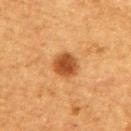Imaged with cross-polarized lighting. The patient is a male in their mid-70s. From the upper back. A close-up tile cropped from a whole-body skin photograph, about 15 mm across. An algorithmic analysis of the crop reported a footprint of about 7 mm², an eccentricity of roughly 0.6, and a symmetry-axis asymmetry near 0.15. And it measured border irregularity of about 1 on a 0–10 scale, a within-lesion color-variation index near 3.5/10, and peripheral color asymmetry of about 1. It also reported an automated nevus-likeness rating near 100 out of 100 and a detector confidence of about 100 out of 100 that the crop contains a lesion.